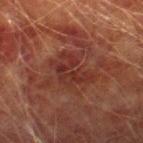biopsy status = catalogued during a skin exam; not biopsied
lighting = cross-polarized illumination
patient = male, in their mid- to late 70s
body site = the right lower leg
automated lesion analysis = an eccentricity of roughly 0.75 and two-axis asymmetry of about 0.4; roughly 6 lightness units darker than nearby skin and a normalized lesion–skin contrast near 6.5; a classifier nevus-likeness of about 0/100 and a lesion-detection confidence of about 100/100
acquisition = total-body-photography crop, ~15 mm field of view